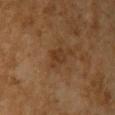workup — no biopsy performed (imaged during a skin exam); patient — female, aged approximately 60; lesion size — about 2.5 mm; illumination — cross-polarized illumination; acquisition — ~15 mm crop, total-body skin-cancer survey; body site — the chest.